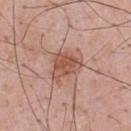| field | value |
|---|---|
| notes | imaged on a skin check; not biopsied |
| subject | male, aged 53–57 |
| location | the upper back |
| illumination | white-light illumination |
| TBP lesion metrics | a footprint of about 8 mm², an outline eccentricity of about 0.6 (0 = round, 1 = elongated), and two-axis asymmetry of about 0.35; a normalized lesion–skin contrast near 7.5; a border-irregularity rating of about 3.5/10 and peripheral color asymmetry of about 1; a detector confidence of about 100 out of 100 that the crop contains a lesion |
| lesion size | about 4 mm |
| image | 15 mm crop, total-body photography |